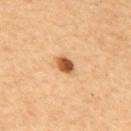Recorded during total-body skin imaging; not selected for excision or biopsy. Cropped from a whole-body photographic skin survey; the tile spans about 15 mm. Longest diameter approximately 2.5 mm. The lesion is located on the mid back. A male patient aged 58–62.